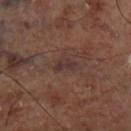notes: no biopsy performed (imaged during a skin exam)
site: the left lower leg
acquisition: ~15 mm crop, total-body skin-cancer survey
automated metrics: an outline eccentricity of about 0.85 (0 = round, 1 = elongated) and a symmetry-axis asymmetry near 0.35; a mean CIELAB color near L≈34 a*≈17 b*≈19, a lesion–skin lightness drop of about 6, and a normalized border contrast of about 7
lighting: cross-polarized illumination
lesion diameter: about 2.5 mm